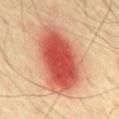Notes:
- notes: catalogued during a skin exam; not biopsied
- automated metrics: a nevus-likeness score of about 100/100 and a lesion-detection confidence of about 100/100
- lighting: cross-polarized
- size: ~9 mm (longest diameter)
- imaging modality: ~15 mm crop, total-body skin-cancer survey
- site: the back
- subject: male, aged approximately 75